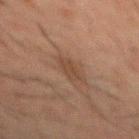{"biopsy_status": "not biopsied; imaged during a skin examination", "image": {"source": "total-body photography crop", "field_of_view_mm": 15}, "automated_metrics": {"cielab_L": 34, "cielab_a": 15, "cielab_b": 24, "vs_skin_darker_L": 6.0, "vs_skin_contrast_norm": 6.0}, "patient": {"sex": "male", "age_approx": 50}, "site": "mid back", "lesion_size": {"long_diameter_mm_approx": 3.0}, "lighting": "cross-polarized"}